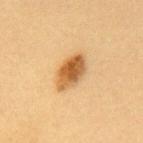The lesion was tiled from a total-body skin photograph and was not biopsied. A region of skin cropped from a whole-body photographic capture, roughly 15 mm wide. A female subject, aged 28 to 32. The recorded lesion diameter is about 4.5 mm. Imaged with cross-polarized lighting. The lesion is on the upper back.